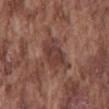The lesion was tiled from a total-body skin photograph and was not biopsied.
This image is a 15 mm lesion crop taken from a total-body photograph.
Longest diameter approximately 4.5 mm.
An algorithmic analysis of the crop reported a border-irregularity index near 3.5/10, internal color variation of about 3.5 on a 0–10 scale, and peripheral color asymmetry of about 1.
From the mid back.
A male subject aged approximately 75.